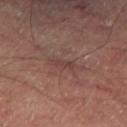imaging modality=15 mm crop, total-body photography; anatomic site=the leg; subject=male, aged around 65.